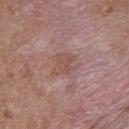Findings:
- workup: imaged on a skin check; not biopsied
- site: the upper back
- image: total-body-photography crop, ~15 mm field of view
- size: ≈3 mm
- automated lesion analysis: a footprint of about 6 mm², a shape eccentricity near 0.45, and a symmetry-axis asymmetry near 0.25; a border-irregularity rating of about 3.5/10, a within-lesion color-variation index near 2/10, and peripheral color asymmetry of about 1; a nevus-likeness score of about 0/100 and a detector confidence of about 100 out of 100 that the crop contains a lesion
- subject: male, aged approximately 50